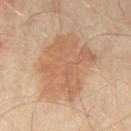notes = imaged on a skin check; not biopsied | lesion diameter = ≈7.5 mm | anatomic site = the left thigh | tile lighting = cross-polarized | patient = about 55 years old | imaging modality = ~15 mm tile from a whole-body skin photo.